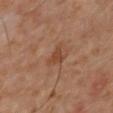{
  "biopsy_status": "not biopsied; imaged during a skin examination",
  "lighting": "cross-polarized",
  "lesion_size": {
    "long_diameter_mm_approx": 2.5
  },
  "image": {
    "source": "total-body photography crop",
    "field_of_view_mm": 15
  },
  "patient": {
    "sex": "male",
    "age_approx": 60
  },
  "site": "back",
  "automated_metrics": {
    "border_irregularity_0_10": 5.0,
    "color_variation_0_10": 2.0,
    "peripheral_color_asymmetry": 0.5
  }
}